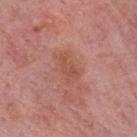Clinical impression:
No biopsy was performed on this lesion — it was imaged during a full skin examination and was not determined to be concerning.
Image and clinical context:
A male patient in their mid- to late 70s. Measured at roughly 3.5 mm in maximum diameter. A roughly 15 mm field-of-view crop from a total-body skin photograph. From the mid back.